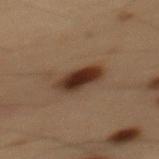Part of a total-body skin-imaging series; this lesion was reviewed on a skin check and was not flagged for biopsy. A lesion tile, about 15 mm wide, cut from a 3D total-body photograph. The patient is a male in their mid-50s. About 4.5 mm across. The lesion is located on the mid back.